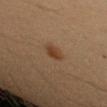biopsy status: imaged on a skin check; not biopsied | lighting: cross-polarized | location: the arm | patient: female, roughly 40 years of age | size: ≈2.5 mm | acquisition: ~15 mm crop, total-body skin-cancer survey.